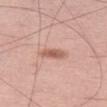{
  "biopsy_status": "not biopsied; imaged during a skin examination",
  "patient": {
    "sex": "male",
    "age_approx": 50
  },
  "lesion_size": {
    "long_diameter_mm_approx": 3.0
  },
  "automated_metrics": {
    "cielab_L": 59,
    "cielab_a": 23,
    "cielab_b": 28,
    "vs_skin_darker_L": 11.0,
    "vs_skin_contrast_norm": 7.5,
    "nevus_likeness_0_100": 75,
    "lesion_detection_confidence_0_100": 100
  },
  "site": "left thigh",
  "image": {
    "source": "total-body photography crop",
    "field_of_view_mm": 15
  }
}